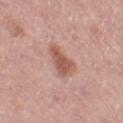| field | value |
|---|---|
| workup | total-body-photography surveillance lesion; no biopsy |
| automated lesion analysis | an eccentricity of roughly 0.85 and a symmetry-axis asymmetry near 0.35; internal color variation of about 3 on a 0–10 scale and radial color variation of about 1 |
| anatomic site | the left thigh |
| patient | female, roughly 50 years of age |
| image source | ~15 mm tile from a whole-body skin photo |
| illumination | white-light illumination |
| size | about 4.5 mm |